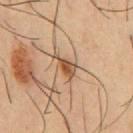<record>
  <biopsy_status>not biopsied; imaged during a skin examination</biopsy_status>
  <site>chest</site>
  <patient>
    <sex>male</sex>
    <age_approx>55</age_approx>
  </patient>
  <lighting>cross-polarized</lighting>
  <image>
    <source>total-body photography crop</source>
    <field_of_view_mm>15</field_of_view_mm>
  </image>
</record>